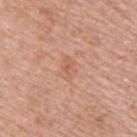biopsy status — total-body-photography surveillance lesion; no biopsy | lesion diameter — ~2.5 mm (longest diameter) | automated metrics — a shape eccentricity near 0.7 and two-axis asymmetry of about 0.25; a lesion color around L≈60 a*≈23 b*≈32 in CIELAB, a lesion–skin lightness drop of about 6, and a lesion-to-skin contrast of about 4.5 (normalized; higher = more distinct); a border-irregularity index near 2.5/10, a color-variation rating of about 2.5/10, and peripheral color asymmetry of about 1 | patient — male, aged 38–42 | image source — ~15 mm crop, total-body skin-cancer survey | body site — the upper back.